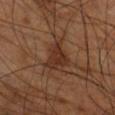workup: no biopsy performed (imaged during a skin exam)
image source: ~15 mm tile from a whole-body skin photo
site: the right lower leg
automated lesion analysis: a symmetry-axis asymmetry near 0.4; border irregularity of about 4 on a 0–10 scale
patient: male, aged 68 to 72
tile lighting: cross-polarized illumination
lesion size: about 4.5 mm